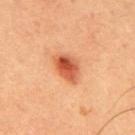Case summary:
* follow-up: catalogued during a skin exam; not biopsied
* automated metrics: a shape eccentricity near 0.75
* subject: male, aged 63–67
* site: the back
* image: ~15 mm tile from a whole-body skin photo
* size: ≈4 mm
* illumination: cross-polarized